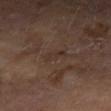Captured during whole-body skin photography for melanoma surveillance; the lesion was not biopsied. The lesion-visualizer software estimated a shape eccentricity near 0.85. Imaged with cross-polarized lighting. Measured at roughly 2.5 mm in maximum diameter. A female patient aged approximately 60. The lesion is located on the arm. A 15 mm close-up extracted from a 3D total-body photography capture.